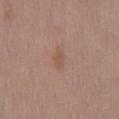Impression:
No biopsy was performed on this lesion — it was imaged during a full skin examination and was not determined to be concerning.